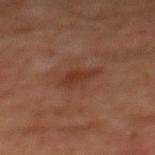The total-body-photography lesion software estimated a lesion area of about 3.5 mm², an outline eccentricity of about 0.9 (0 = round, 1 = elongated), and two-axis asymmetry of about 0.35. It also reported about 7 CIELAB-L* units darker than the surrounding skin and a lesion-to-skin contrast of about 7 (normalized; higher = more distinct). And it measured a border-irregularity rating of about 4/10, internal color variation of about 1 on a 0–10 scale, and a peripheral color-asymmetry measure near 0.5. A 15 mm crop from a total-body photograph taken for skin-cancer surveillance. The subject is a male roughly 60 years of age. Measured at roughly 3 mm in maximum diameter. The lesion is located on the chest. Imaged with cross-polarized lighting.